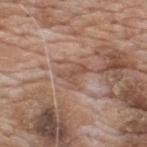{
  "biopsy_status": "not biopsied; imaged during a skin examination",
  "image": {
    "source": "total-body photography crop",
    "field_of_view_mm": 15
  },
  "site": "upper back",
  "automated_metrics": {
    "cielab_L": 51,
    "cielab_a": 19,
    "cielab_b": 27,
    "vs_skin_darker_L": 8.0,
    "vs_skin_contrast_norm": 6.0
  },
  "lesion_size": {
    "long_diameter_mm_approx": 3.5
  },
  "patient": {
    "sex": "male",
    "age_approx": 60
  }
}